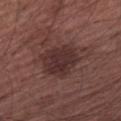workup — imaged on a skin check; not biopsied
lighting — white-light illumination
automated lesion analysis — a footprint of about 14 mm², an eccentricity of roughly 0.6, and a shape-asymmetry score of about 0.2 (0 = symmetric); a border-irregularity rating of about 2.5/10, internal color variation of about 2.5 on a 0–10 scale, and peripheral color asymmetry of about 1
imaging modality — 15 mm crop, total-body photography
patient — male, approximately 65 years of age
site — the left upper arm
size — ≈4.5 mm